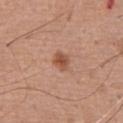No biopsy was performed on this lesion — it was imaged during a full skin examination and was not determined to be concerning. The lesion is on the front of the torso. A 15 mm close-up extracted from a 3D total-body photography capture. The subject is a male approximately 75 years of age. The lesion's longest dimension is about 2.5 mm.